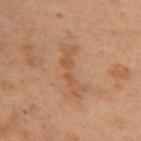Impression:
The lesion was photographed on a routine skin check and not biopsied; there is no pathology result.
Acquisition and patient details:
The lesion's longest dimension is about 5.5 mm. A lesion tile, about 15 mm wide, cut from a 3D total-body photograph. A female patient, in their mid-50s. An algorithmic analysis of the crop reported an average lesion color of about L≈44 a*≈18 b*≈29 (CIELAB) and a lesion–skin lightness drop of about 6. The analysis additionally found a color-variation rating of about 1.5/10 and peripheral color asymmetry of about 0. On the back. This is a cross-polarized tile.